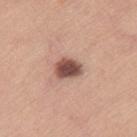This lesion was catalogued during total-body skin photography and was not selected for biopsy. A female subject aged 58–62. About 3 mm across. The tile uses white-light illumination. A 15 mm close-up tile from a total-body photography series done for melanoma screening. The lesion is on the right thigh.